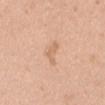Cropped from a whole-body photographic skin survey; the tile spans about 15 mm. Located on the front of the torso. The total-body-photography lesion software estimated roughly 7 lightness units darker than nearby skin and a lesion-to-skin contrast of about 5 (normalized; higher = more distinct). The analysis additionally found border irregularity of about 5.5 on a 0–10 scale, a within-lesion color-variation index near 0.5/10, and a peripheral color-asymmetry measure near 0. And it measured an automated nevus-likeness rating near 0 out of 100. A female subject, about 40 years old. About 3.5 mm across.